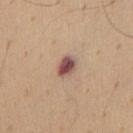Recorded during total-body skin imaging; not selected for excision or biopsy. The lesion is on the chest. The tile uses white-light illumination. The recorded lesion diameter is about 2.5 mm. A male patient, in their mid- to late 50s. A 15 mm crop from a total-body photograph taken for skin-cancer surveillance.